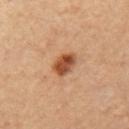biopsy status: total-body-photography surveillance lesion; no biopsy | anatomic site: the left upper arm | tile lighting: cross-polarized illumination | lesion size: ~3 mm (longest diameter) | patient: female, aged around 45 | image: ~15 mm tile from a whole-body skin photo | image-analysis metrics: border irregularity of about 1.5 on a 0–10 scale and a color-variation rating of about 4.5/10; a nevus-likeness score of about 95/100 and a lesion-detection confidence of about 100/100.